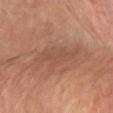Findings:
– image source — ~15 mm tile from a whole-body skin photo
– automated metrics — a footprint of about 12 mm², a shape eccentricity near 0.9, and a symmetry-axis asymmetry near 0.35
– lesion size — about 6.5 mm
– patient — female, about 65 years old
– site — the chest
– illumination — cross-polarized illumination